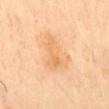• workup · total-body-photography surveillance lesion; no biopsy
• diameter · about 4.5 mm
• subject · male, aged around 50
• anatomic site · the mid back
• imaging modality · ~15 mm crop, total-body skin-cancer survey